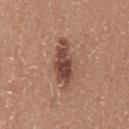Captured during whole-body skin photography for melanoma surveillance; the lesion was not biopsied.